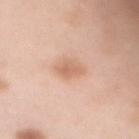This lesion was catalogued during total-body skin photography and was not selected for biopsy. The tile uses white-light illumination. Cropped from a total-body skin-imaging series; the visible field is about 15 mm. The lesion is on the mid back. The lesion-visualizer software estimated a border-irregularity rating of about 3.5/10, a color-variation rating of about 2/10, and peripheral color asymmetry of about 0.5. The analysis additionally found an automated nevus-likeness rating near 75 out of 100 and a lesion-detection confidence of about 100/100. The subject is a female aged approximately 50. The lesion's longest dimension is about 3.5 mm.